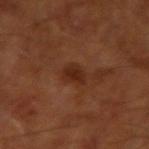size: about 3 mm
tile lighting: cross-polarized
TBP lesion metrics: an outline eccentricity of about 0.8 (0 = round, 1 = elongated) and two-axis asymmetry of about 0.25; a mean CIELAB color near L≈26 a*≈21 b*≈28, about 7 CIELAB-L* units darker than the surrounding skin, and a lesion-to-skin contrast of about 8 (normalized; higher = more distinct)
location: the arm
subject: male, roughly 65 years of age
acquisition: ~15 mm crop, total-body skin-cancer survey